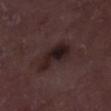• acquisition · 15 mm crop, total-body photography
• image-analysis metrics · an area of roughly 11 mm², a shape eccentricity near 0.85, and a symmetry-axis asymmetry near 0.3; a mean CIELAB color near L≈20 a*≈15 b*≈13, a lesion–skin lightness drop of about 9, and a normalized border contrast of about 10.5; border irregularity of about 3.5 on a 0–10 scale, a color-variation rating of about 6/10, and peripheral color asymmetry of about 2
• anatomic site · the right lower leg
• patient · male, in their 70s
• biopsy diagnosis · a seborrheic keratosis (benign)